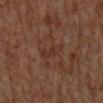The lesion was photographed on a routine skin check and not biopsied; there is no pathology result. Automated tile analysis of the lesion measured a mean CIELAB color near L≈24 a*≈14 b*≈20 and a normalized border contrast of about 5. It also reported border irregularity of about 4.5 on a 0–10 scale, internal color variation of about 1.5 on a 0–10 scale, and a peripheral color-asymmetry measure near 0.5. The software also gave a classifier nevus-likeness of about 0/100 and a lesion-detection confidence of about 95/100. A 15 mm close-up extracted from a 3D total-body photography capture. The patient is a male about 80 years old. From the mid back. This is a cross-polarized tile.